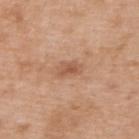Assessment: Recorded during total-body skin imaging; not selected for excision or biopsy. Context: The tile uses white-light illumination. A lesion tile, about 15 mm wide, cut from a 3D total-body photograph. The lesion is on the upper back. The patient is a male aged 48 to 52. Measured at roughly 3 mm in maximum diameter.